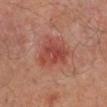Clinical impression: The lesion was photographed on a routine skin check and not biopsied; there is no pathology result. Clinical summary: The lesion is located on the left leg. A 15 mm close-up extracted from a 3D total-body photography capture. A male patient aged around 50.